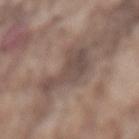A male patient aged approximately 75.
Captured under white-light illumination.
From the lower back.
A close-up tile cropped from a whole-body skin photograph, about 15 mm across.
The recorded lesion diameter is about 5 mm.